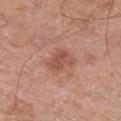Clinical impression:
No biopsy was performed on this lesion — it was imaged during a full skin examination and was not determined to be concerning.
Acquisition and patient details:
From the right lower leg. A male subject, about 60 years old. The lesion-visualizer software estimated a color-variation rating of about 2/10 and peripheral color asymmetry of about 0.5. It also reported a nevus-likeness score of about 20/100. A close-up tile cropped from a whole-body skin photograph, about 15 mm across.